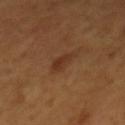subject — male, roughly 45 years of age | imaging modality — total-body-photography crop, ~15 mm field of view | lesion diameter — about 3 mm | location — the mid back | automated metrics — an eccentricity of roughly 0.85 and two-axis asymmetry of about 0.35; a lesion color around L≈30 a*≈20 b*≈28 in CIELAB, about 7 CIELAB-L* units darker than the surrounding skin, and a lesion-to-skin contrast of about 7 (normalized; higher = more distinct).